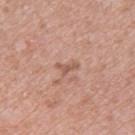| key | value |
|---|---|
| location | the upper back |
| lesion size | ~2.5 mm (longest diameter) |
| patient | female, roughly 40 years of age |
| acquisition | 15 mm crop, total-body photography |
| image-analysis metrics | an average lesion color of about L≈56 a*≈22 b*≈28 (CIELAB), about 9 CIELAB-L* units darker than the surrounding skin, and a normalized lesion–skin contrast near 6 |
| lighting | white-light |